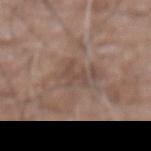Case summary:
– workup · catalogued during a skin exam; not biopsied
– site · the abdomen
– illumination · white-light
– imaging modality · ~15 mm tile from a whole-body skin photo
– lesion diameter · ≈3.5 mm
– patient · male, aged 73 to 77